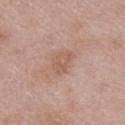biopsy status=catalogued during a skin exam; not biopsied
size=~3.5 mm (longest diameter)
lighting=white-light illumination
body site=the lower back
image source=15 mm crop, total-body photography
subject=male, aged around 50